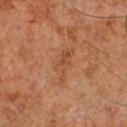follow-up — total-body-photography surveillance lesion; no biopsy | site — the front of the torso | lesion diameter — ~4.5 mm (longest diameter) | automated metrics — a mean CIELAB color near L≈47 a*≈23 b*≈35 and a normalized lesion–skin contrast near 5.5; a classifier nevus-likeness of about 0/100 and a detector confidence of about 100 out of 100 that the crop contains a lesion | illumination — cross-polarized illumination | image source — ~15 mm tile from a whole-body skin photo | subject — male, aged approximately 60.